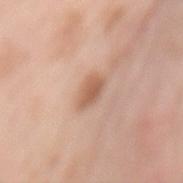Captured during whole-body skin photography for melanoma surveillance; the lesion was not biopsied. The total-body-photography lesion software estimated a mean CIELAB color near L≈60 a*≈21 b*≈31 and a normalized border contrast of about 7. The analysis additionally found a nevus-likeness score of about 70/100. Imaged with white-light lighting. The patient is a female about 50 years old. From the mid back. A roughly 15 mm field-of-view crop from a total-body skin photograph. The recorded lesion diameter is about 3.5 mm.